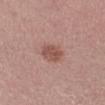follow-up: total-body-photography surveillance lesion; no biopsy | body site: the arm | lighting: white-light | acquisition: total-body-photography crop, ~15 mm field of view | automated metrics: an average lesion color of about L≈52 a*≈22 b*≈24 (CIELAB), a lesion–skin lightness drop of about 10, and a normalized lesion–skin contrast near 7; border irregularity of about 1.5 on a 0–10 scale and peripheral color asymmetry of about 1; an automated nevus-likeness rating near 60 out of 100 and a lesion-detection confidence of about 100/100 | patient: female, aged around 25 | size: about 3 mm.